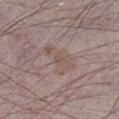Captured during whole-body skin photography for melanoma surveillance; the lesion was not biopsied.